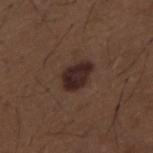Assessment: The lesion was photographed on a routine skin check and not biopsied; there is no pathology result. Clinical summary: A male patient, aged approximately 50. The tile uses white-light illumination. Longest diameter approximately 3.5 mm. From the mid back. A 15 mm close-up tile from a total-body photography series done for melanoma screening.